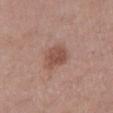The patient is a female aged 48–52. The tile uses white-light illumination. Approximately 3.5 mm at its widest. From the right lower leg. Cropped from a whole-body photographic skin survey; the tile spans about 15 mm.